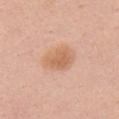notes — imaged on a skin check; not biopsied | anatomic site — the chest | image-analysis metrics — a lesion area of about 10 mm², an outline eccentricity of about 0.65 (0 = round, 1 = elongated), and a symmetry-axis asymmetry near 0.2; an average lesion color of about L≈64 a*≈21 b*≈34 (CIELAB), a lesion–skin lightness drop of about 9, and a lesion-to-skin contrast of about 6.5 (normalized; higher = more distinct); a border-irregularity index near 2/10, internal color variation of about 3 on a 0–10 scale, and a peripheral color-asymmetry measure near 1 | subject — female, in their 40s | acquisition — ~15 mm crop, total-body skin-cancer survey | diameter — ≈4 mm | lighting — white-light illumination.